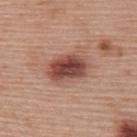Q: Was a biopsy performed?
A: imaged on a skin check; not biopsied
Q: Where on the body is the lesion?
A: the upper back
Q: What are the patient's age and sex?
A: female, approximately 60 years of age
Q: What kind of image is this?
A: total-body-photography crop, ~15 mm field of view
Q: How was the tile lit?
A: white-light
Q: What is the lesion's diameter?
A: ~5 mm (longest diameter)